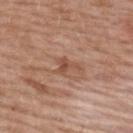Impression: No biopsy was performed on this lesion — it was imaged during a full skin examination and was not determined to be concerning. Background: This is a white-light tile. A female subject aged approximately 60. A close-up tile cropped from a whole-body skin photograph, about 15 mm across. From the upper back. An algorithmic analysis of the crop reported two-axis asymmetry of about 0.65. The software also gave an automated nevus-likeness rating near 0 out of 100 and a detector confidence of about 100 out of 100 that the crop contains a lesion.